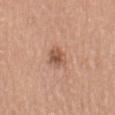| feature | finding |
|---|---|
| biopsy status | catalogued during a skin exam; not biopsied |
| illumination | white-light |
| patient | female, aged approximately 70 |
| body site | the right thigh |
| image | 15 mm crop, total-body photography |